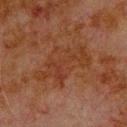| key | value |
|---|---|
| imaging modality | ~15 mm tile from a whole-body skin photo |
| lesion diameter | ~7 mm (longest diameter) |
| patient | male, aged around 80 |
| site | the upper back |
| tile lighting | cross-polarized illumination |